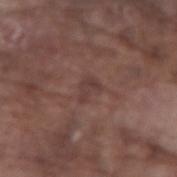{
  "biopsy_status": "not biopsied; imaged during a skin examination",
  "site": "right forearm",
  "lighting": "white-light",
  "image": {
    "source": "total-body photography crop",
    "field_of_view_mm": 15
  },
  "patient": {
    "sex": "male",
    "age_approx": 75
  },
  "lesion_size": {
    "long_diameter_mm_approx": 2.5
  }
}